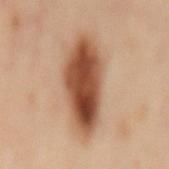This lesion was catalogued during total-body skin photography and was not selected for biopsy. The recorded lesion diameter is about 9.5 mm. The lesion is located on the mid back. A female patient in their 60s. A roughly 15 mm field-of-view crop from a total-body skin photograph. Captured under cross-polarized illumination.